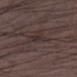size = about 3.5 mm; image = total-body-photography crop, ~15 mm field of view; location = the left lower leg; tile lighting = white-light illumination; subject = male, aged around 50; automated lesion analysis = a classifier nevus-likeness of about 0/100.